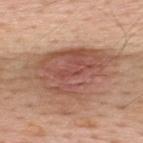The lesion was photographed on a routine skin check and not biopsied; there is no pathology result.
The lesion-visualizer software estimated an average lesion color of about L≈53 a*≈22 b*≈28 (CIELAB), a lesion–skin lightness drop of about 13, and a normalized lesion–skin contrast near 9.
The patient is a male approximately 65 years of age.
Cropped from a total-body skin-imaging series; the visible field is about 15 mm.
On the upper back.
Imaged with white-light lighting.
Measured at roughly 10.5 mm in maximum diameter.